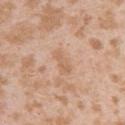  biopsy_status: not biopsied; imaged during a skin examination
  patient:
    sex: female
    age_approx: 25
  image:
    source: total-body photography crop
    field_of_view_mm: 15
  lighting: white-light
  lesion_size:
    long_diameter_mm_approx: 3.5
  site: left upper arm